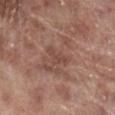Impression:
Imaged during a routine full-body skin examination; the lesion was not biopsied and no histopathology is available.
Clinical summary:
Imaged with white-light lighting. Automated tile analysis of the lesion measured a lesion area of about 4 mm², an outline eccentricity of about 0.85 (0 = round, 1 = elongated), and two-axis asymmetry of about 0.55. The software also gave a color-variation rating of about 0/10 and a peripheral color-asymmetry measure near 0. The analysis additionally found a lesion-detection confidence of about 100/100. From the leg. The lesion's longest dimension is about 3 mm. A roughly 15 mm field-of-view crop from a total-body skin photograph. A male patient aged 68–72.